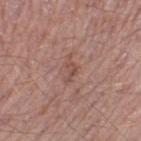- notes: total-body-photography surveillance lesion; no biopsy
- patient: male, approximately 50 years of age
- tile lighting: white-light
- automated metrics: a mean CIELAB color near L≈49 a*≈21 b*≈25, a lesion–skin lightness drop of about 7, and a lesion-to-skin contrast of about 5.5 (normalized; higher = more distinct); a border-irregularity rating of about 6/10 and internal color variation of about 0 on a 0–10 scale
- imaging modality: total-body-photography crop, ~15 mm field of view
- diameter: ≈3 mm
- anatomic site: the left thigh